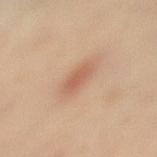Clinical impression: Captured during whole-body skin photography for melanoma surveillance; the lesion was not biopsied. Image and clinical context: A 15 mm close-up tile from a total-body photography series done for melanoma screening. The lesion is located on the right leg. The tile uses cross-polarized illumination. About 3 mm across. A female patient, aged 38 to 42.